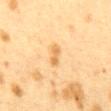Impression: The lesion was photographed on a routine skin check and not biopsied; there is no pathology result. Clinical summary: A lesion tile, about 15 mm wide, cut from a 3D total-body photograph. The lesion is located on the mid back. The tile uses cross-polarized illumination. Automated image analysis of the tile measured a footprint of about 3.5 mm², an eccentricity of roughly 0.9, and two-axis asymmetry of about 0.25. And it measured a lesion–skin lightness drop of about 8 and a lesion-to-skin contrast of about 6.5 (normalized; higher = more distinct). The analysis additionally found border irregularity of about 2.5 on a 0–10 scale and internal color variation of about 1.5 on a 0–10 scale. Longest diameter approximately 3 mm. A female subject, aged around 40.